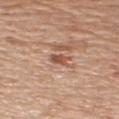  biopsy_status: not biopsied; imaged during a skin examination
  patient:
    sex: male
    age_approx: 65
  site: right upper arm
  image:
    source: total-body photography crop
    field_of_view_mm: 15